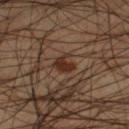The lesion was photographed on a routine skin check and not biopsied; there is no pathology result. A male patient aged 43 to 47. Captured under cross-polarized illumination. Located on the left thigh. A region of skin cropped from a whole-body photographic capture, roughly 15 mm wide. An algorithmic analysis of the crop reported an area of roughly 4.5 mm², an outline eccentricity of about 0.4 (0 = round, 1 = elongated), and a shape-asymmetry score of about 0.3 (0 = symmetric). And it measured a lesion color around L≈29 a*≈17 b*≈25 in CIELAB and roughly 8 lightness units darker than nearby skin. And it measured a border-irregularity rating of about 3.5/10 and a within-lesion color-variation index near 1.5/10.